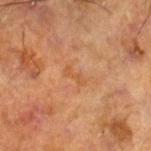notes = catalogued during a skin exam; not biopsied
tile lighting = cross-polarized illumination
location = the right lower leg
patient = male, aged 68 to 72
imaging modality = ~15 mm tile from a whole-body skin photo